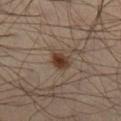Clinical impression: Recorded during total-body skin imaging; not selected for excision or biopsy.